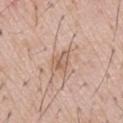The lesion was photographed on a routine skin check and not biopsied; there is no pathology result.
This is a white-light tile.
Cropped from a total-body skin-imaging series; the visible field is about 15 mm.
A male subject approximately 55 years of age.
About 2.5 mm across.
The lesion-visualizer software estimated a lesion area of about 4 mm² and a symmetry-axis asymmetry near 0.4. And it measured a lesion color around L≈60 a*≈18 b*≈29 in CIELAB, a lesion–skin lightness drop of about 8, and a lesion-to-skin contrast of about 6 (normalized; higher = more distinct). And it measured a classifier nevus-likeness of about 0/100 and a detector confidence of about 95 out of 100 that the crop contains a lesion.